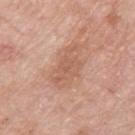Q: Is there a histopathology result?
A: catalogued during a skin exam; not biopsied
Q: How was this image acquired?
A: 15 mm crop, total-body photography
Q: Lesion location?
A: the right upper arm
Q: What are the patient's age and sex?
A: female, roughly 65 years of age